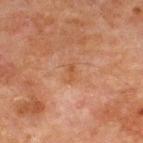notes — no biopsy performed (imaged during a skin exam) | lesion size — ≈2.5 mm | automated metrics — an average lesion color of about L≈43 a*≈19 b*≈30 (CIELAB), about 5 CIELAB-L* units darker than the surrounding skin, and a normalized border contrast of about 5; an automated nevus-likeness rating near 0 out of 100 and lesion-presence confidence of about 100/100 | tile lighting — cross-polarized illumination | image source — ~15 mm crop, total-body skin-cancer survey | patient — male, aged 63–67 | anatomic site — the front of the torso.